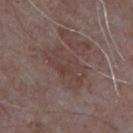Part of a total-body skin-imaging series; this lesion was reviewed on a skin check and was not flagged for biopsy. The lesion is located on the left upper arm. The recorded lesion diameter is about 5.5 mm. This is a white-light tile. A roughly 15 mm field-of-view crop from a total-body skin photograph. A male patient in their mid- to late 60s.